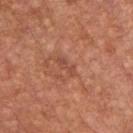Imaged during a routine full-body skin examination; the lesion was not biopsied and no histopathology is available. From the chest. Imaged with white-light lighting. A male patient about 65 years old. A 15 mm crop from a total-body photograph taken for skin-cancer surveillance.